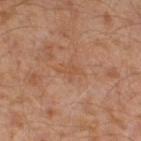<tbp_lesion>
  <site>left leg</site>
  <automated_metrics>
    <cielab_L>50</cielab_L>
    <cielab_a>21</cielab_a>
    <cielab_b>33</cielab_b>
    <vs_skin_darker_L>5.0</vs_skin_darker_L>
    <vs_skin_contrast_norm>4.5</vs_skin_contrast_norm>
    <nevus_likeness_0_100>0</nevus_likeness_0_100>
  </automated_metrics>
  <lighting>cross-polarized</lighting>
  <lesion_size>
    <long_diameter_mm_approx>2.5</long_diameter_mm_approx>
  </lesion_size>
  <patient>
    <sex>male</sex>
    <age_approx>30</age_approx>
  </patient>
  <image>
    <source>total-body photography crop</source>
    <field_of_view_mm>15</field_of_view_mm>
  </image>
</tbp_lesion>